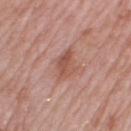Q: Was this lesion biopsied?
A: no biopsy performed (imaged during a skin exam)
Q: Patient demographics?
A: female, aged approximately 70
Q: Lesion size?
A: ≈3.5 mm
Q: How was this image acquired?
A: ~15 mm crop, total-body skin-cancer survey
Q: Lesion location?
A: the leg